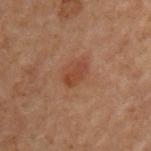{"patient": {"sex": "male", "age_approx": 70}, "site": "chest", "image": {"source": "total-body photography crop", "field_of_view_mm": 15}}